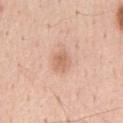Assessment:
Part of a total-body skin-imaging series; this lesion was reviewed on a skin check and was not flagged for biopsy.
Background:
A male patient aged approximately 55. Captured under white-light illumination. A 15 mm close-up tile from a total-body photography series done for melanoma screening. Automated tile analysis of the lesion measured a footprint of about 5 mm² and an outline eccentricity of about 0.7 (0 = round, 1 = elongated). The software also gave border irregularity of about 2.5 on a 0–10 scale, a color-variation rating of about 2/10, and a peripheral color-asymmetry measure near 0.5. The lesion's longest dimension is about 3 mm. The lesion is on the chest.